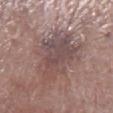{"image": {"source": "total-body photography crop", "field_of_view_mm": 15}, "site": "left lower leg", "patient": {"sex": "male", "age_approx": 70}}